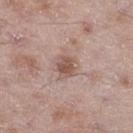Recorded during total-body skin imaging; not selected for excision or biopsy. A 15 mm close-up extracted from a 3D total-body photography capture. A male subject, aged 48–52. The lesion is on the right thigh. Measured at roughly 2.5 mm in maximum diameter. The tile uses white-light illumination. The total-body-photography lesion software estimated a lesion area of about 5 mm² and an outline eccentricity of about 0.5 (0 = round, 1 = elongated). It also reported roughly 11 lightness units darker than nearby skin and a lesion-to-skin contrast of about 7.5 (normalized; higher = more distinct).